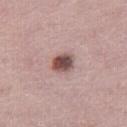biopsy status = imaged on a skin check; not biopsied
body site = the leg
automated lesion analysis = a mean CIELAB color near L≈49 a*≈20 b*≈21, roughly 17 lightness units darker than nearby skin, and a normalized border contrast of about 11.5; lesion-presence confidence of about 100/100
subject = female, aged 48 to 52
lesion diameter = about 2.5 mm
acquisition = ~15 mm tile from a whole-body skin photo
illumination = white-light illumination